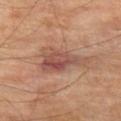biopsy status: catalogued during a skin exam; not biopsied | body site: the left thigh | subject: male, roughly 70 years of age | size: about 6.5 mm | imaging modality: total-body-photography crop, ~15 mm field of view | tile lighting: cross-polarized | automated metrics: an area of roughly 13 mm² and a shape eccentricity near 0.9; a color-variation rating of about 7/10 and a peripheral color-asymmetry measure near 2.